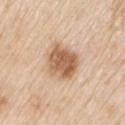The lesion was photographed on a routine skin check and not biopsied; there is no pathology result. This image is a 15 mm lesion crop taken from a total-body photograph. The lesion is on the right upper arm. A male subject, roughly 80 years of age. The recorded lesion diameter is about 4 mm. Automated image analysis of the tile measured a lesion-detection confidence of about 100/100.